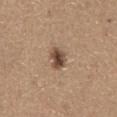Imaged during a routine full-body skin examination; the lesion was not biopsied and no histopathology is available.
The patient is a male in their mid-60s.
Imaged with white-light lighting.
A lesion tile, about 15 mm wide, cut from a 3D total-body photograph.
Approximately 3 mm at its widest.
Located on the abdomen.